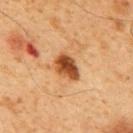This is a cross-polarized tile.
The lesion's longest dimension is about 3.5 mm.
A male subject, aged 58 to 62.
The lesion is located on the mid back.
A region of skin cropped from a whole-body photographic capture, roughly 15 mm wide.
The lesion-visualizer software estimated a footprint of about 7 mm², an outline eccentricity of about 0.75 (0 = round, 1 = elongated), and a shape-asymmetry score of about 0.25 (0 = symmetric). And it measured a mean CIELAB color near L≈41 a*≈23 b*≈35, about 15 CIELAB-L* units darker than the surrounding skin, and a lesion-to-skin contrast of about 12 (normalized; higher = more distinct). The software also gave border irregularity of about 2.5 on a 0–10 scale, a color-variation rating of about 5/10, and peripheral color asymmetry of about 1.5. And it measured a nevus-likeness score of about 95/100 and lesion-presence confidence of about 100/100.